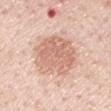notes: no biopsy performed (imaged during a skin exam)
image: ~15 mm crop, total-body skin-cancer survey
subject: male, aged around 60
anatomic site: the right upper arm
size: ≈6.5 mm
illumination: white-light illumination
automated lesion analysis: an outline eccentricity of about 0.5 (0 = round, 1 = elongated) and a shape-asymmetry score of about 0.15 (0 = symmetric); an average lesion color of about L≈67 a*≈21 b*≈30 (CIELAB) and roughly 11 lightness units darker than nearby skin; a border-irregularity index near 2/10, internal color variation of about 3.5 on a 0–10 scale, and peripheral color asymmetry of about 1.5